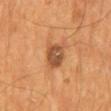Findings:
* notes: imaged on a skin check; not biopsied
* anatomic site: the mid back
* patient: male, in their mid-50s
* acquisition: total-body-photography crop, ~15 mm field of view